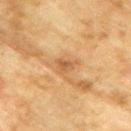Impression:
Recorded during total-body skin imaging; not selected for excision or biopsy.
Background:
The tile uses cross-polarized illumination. The patient is a male aged approximately 75. An algorithmic analysis of the crop reported a footprint of about 3.5 mm² and an eccentricity of roughly 0.7. The software also gave border irregularity of about 3 on a 0–10 scale, a color-variation rating of about 3/10, and peripheral color asymmetry of about 1. This image is a 15 mm lesion crop taken from a total-body photograph. Longest diameter approximately 2.5 mm. From the back.